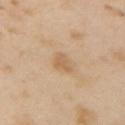The lesion was photographed on a routine skin check and not biopsied; there is no pathology result. Longest diameter approximately 2.5 mm. A 15 mm crop from a total-body photograph taken for skin-cancer surveillance. A female patient, aged around 40. Located on the left arm. The total-body-photography lesion software estimated a footprint of about 3 mm², an outline eccentricity of about 0.7 (0 = round, 1 = elongated), and a shape-asymmetry score of about 0.45 (0 = symmetric). And it measured a lesion color around L≈61 a*≈18 b*≈36 in CIELAB, about 8 CIELAB-L* units darker than the surrounding skin, and a normalized lesion–skin contrast near 5.5.